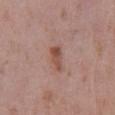Q: Is there a histopathology result?
A: total-body-photography surveillance lesion; no biopsy